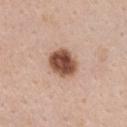Q: Was this lesion biopsied?
A: imaged on a skin check; not biopsied
Q: Lesion size?
A: ≈4 mm
Q: What is the imaging modality?
A: 15 mm crop, total-body photography
Q: What are the patient's age and sex?
A: female, aged 33–37
Q: How was the tile lit?
A: white-light illumination
Q: Lesion location?
A: the chest